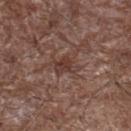biopsy status: no biopsy performed (imaged during a skin exam) | image: 15 mm crop, total-body photography | lesion diameter: ≈3 mm | patient: male, aged around 70 | site: the left thigh | automated lesion analysis: an area of roughly 4.5 mm², an outline eccentricity of about 0.75 (0 = round, 1 = elongated), and a shape-asymmetry score of about 0.4 (0 = symmetric); about 8 CIELAB-L* units darker than the surrounding skin and a normalized lesion–skin contrast near 6.5; an automated nevus-likeness rating near 0 out of 100 and lesion-presence confidence of about 95/100.